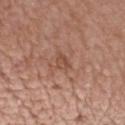Q: Was a biopsy performed?
A: imaged on a skin check; not biopsied
Q: What lighting was used for the tile?
A: white-light
Q: What is the anatomic site?
A: the chest
Q: How large is the lesion?
A: ~2.5 mm (longest diameter)
Q: What are the patient's age and sex?
A: male, in their 50s
Q: How was this image acquired?
A: 15 mm crop, total-body photography